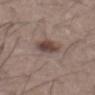biopsy status: imaged on a skin check; not biopsied
location: the abdomen
image-analysis metrics: an automated nevus-likeness rating near 95 out of 100
imaging modality: ~15 mm tile from a whole-body skin photo
diameter: about 3.5 mm
patient: male, in their mid- to late 50s